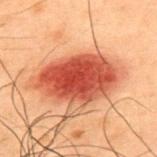The lesion was tiled from a total-body skin photograph and was not biopsied.
This image is a 15 mm lesion crop taken from a total-body photograph.
On the upper back.
A male patient approximately 50 years of age.
Approximately 7.5 mm at its widest.